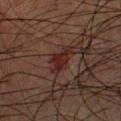Case summary:
- workup: imaged on a skin check; not biopsied
- automated lesion analysis: a footprint of about 4 mm², a shape eccentricity near 0.85, and two-axis asymmetry of about 0.35
- image source: ~15 mm tile from a whole-body skin photo
- lesion diameter: ~3 mm (longest diameter)
- subject: male, aged 33 to 37
- site: the chest